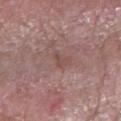acquisition: 15 mm crop, total-body photography
site: the right forearm
patient: male, about 60 years old
diameter: ~2.5 mm (longest diameter)